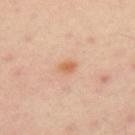<tbp_lesion>
<automated_metrics>
  <nevus_likeness_0_100>50</nevus_likeness_0_100>
  <lesion_detection_confidence_0_100>100</lesion_detection_confidence_0_100>
</automated_metrics>
<lesion_size>
  <long_diameter_mm_approx>2.0</long_diameter_mm_approx>
</lesion_size>
<site>left upper arm</site>
<patient>
  <sex>male</sex>
  <age_approx>55</age_approx>
</patient>
<image>
  <source>total-body photography crop</source>
  <field_of_view_mm>15</field_of_view_mm>
</image>
</tbp_lesion>